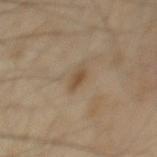• biopsy status · total-body-photography surveillance lesion; no biopsy
• size · ~3 mm (longest diameter)
• patient · male, in their mid- to late 50s
• image · ~15 mm tile from a whole-body skin photo
• site · the mid back
• illumination · cross-polarized